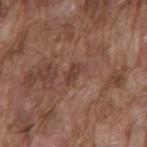| field | value |
|---|---|
| follow-up | catalogued during a skin exam; not biopsied |
| image source | total-body-photography crop, ~15 mm field of view |
| subject | male, approximately 75 years of age |
| body site | the mid back |